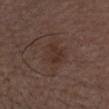No biopsy was performed on this lesion — it was imaged during a full skin examination and was not determined to be concerning. A close-up tile cropped from a whole-body skin photograph, about 15 mm across. The lesion is located on the head or neck. A male patient, aged approximately 75.